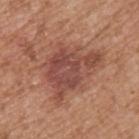follow-up = imaged on a skin check; not biopsied
automated metrics = a lesion area of about 25 mm², a shape eccentricity near 0.75, and two-axis asymmetry of about 0.4; a color-variation rating of about 4/10 and radial color variation of about 1; an automated nevus-likeness rating near 35 out of 100 and a detector confidence of about 100 out of 100 that the crop contains a lesion
image = 15 mm crop, total-body photography
subject = male, aged approximately 55
site = the left upper arm